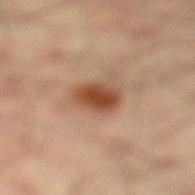Assessment: Imaged during a routine full-body skin examination; the lesion was not biopsied and no histopathology is available. Context: Automated tile analysis of the lesion measured a lesion color around L≈43 a*≈21 b*≈31 in CIELAB, roughly 13 lightness units darker than nearby skin, and a normalized border contrast of about 10.5. And it measured a classifier nevus-likeness of about 100/100. Measured at roughly 3.5 mm in maximum diameter. A male patient, aged around 35. A 15 mm close-up extracted from a 3D total-body photography capture. From the left lower leg.